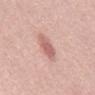location = the mid back
automated metrics = a footprint of about 6.5 mm², a shape eccentricity near 0.75, and two-axis asymmetry of about 0.2; a lesion color around L≈62 a*≈24 b*≈25 in CIELAB and a lesion–skin lightness drop of about 11
diameter = ~3.5 mm (longest diameter)
subject = female, about 55 years old
image source = total-body-photography crop, ~15 mm field of view